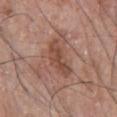biopsy status = imaged on a skin check; not biopsied
acquisition = total-body-photography crop, ~15 mm field of view
diameter = ~5.5 mm (longest diameter)
site = the chest
patient = male, in their 70s